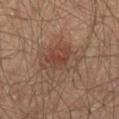Clinical impression: Imaged during a routine full-body skin examination; the lesion was not biopsied and no histopathology is available. Image and clinical context: Imaged with cross-polarized lighting. A male patient, approximately 65 years of age. A close-up tile cropped from a whole-body skin photograph, about 15 mm across. The lesion-visualizer software estimated an outline eccentricity of about 0.55 (0 = round, 1 = elongated) and a shape-asymmetry score of about 0.3 (0 = symmetric). And it measured a nevus-likeness score of about 75/100 and a lesion-detection confidence of about 100/100. On the left thigh. Longest diameter approximately 5 mm.